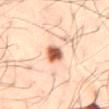Impression:
Imaged during a routine full-body skin examination; the lesion was not biopsied and no histopathology is available.
Context:
The recorded lesion diameter is about 3 mm. A male subject aged 48 to 52. A region of skin cropped from a whole-body photographic capture, roughly 15 mm wide. Captured under cross-polarized illumination. The lesion is on the mid back.